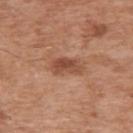The lesion was photographed on a routine skin check and not biopsied; there is no pathology result. Imaged with white-light lighting. Located on the right upper arm. A roughly 15 mm field-of-view crop from a total-body skin photograph. Approximately 3.5 mm at its widest. A male patient, in their mid-60s.